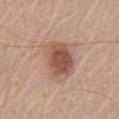Captured during whole-body skin photography for melanoma surveillance; the lesion was not biopsied. A male subject aged 68 to 72. About 5.5 mm across. This is a white-light tile. Automated tile analysis of the lesion measured a border-irregularity rating of about 2/10 and peripheral color asymmetry of about 1.5. It also reported lesion-presence confidence of about 100/100. This image is a 15 mm lesion crop taken from a total-body photograph. On the front of the torso.